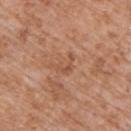Imaged during a routine full-body skin examination; the lesion was not biopsied and no histopathology is available.
Cropped from a whole-body photographic skin survey; the tile spans about 15 mm.
A male patient in their 60s.
The lesion is located on the back.